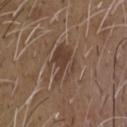workup — no biopsy performed (imaged during a skin exam)
patient — male, aged around 50
illumination — white-light
TBP lesion metrics — a classifier nevus-likeness of about 0/100
body site — the chest
acquisition — ~15 mm crop, total-body skin-cancer survey
diameter — ~4.5 mm (longest diameter)